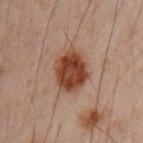Part of a total-body skin-imaging series; this lesion was reviewed on a skin check and was not flagged for biopsy.
A male patient, about 50 years old.
From the left upper arm.
This is a cross-polarized tile.
A 15 mm crop from a total-body photograph taken for skin-cancer surveillance.
An algorithmic analysis of the crop reported a footprint of about 14 mm². The software also gave a mean CIELAB color near L≈34 a*≈19 b*≈26, about 12 CIELAB-L* units darker than the surrounding skin, and a lesion-to-skin contrast of about 11.5 (normalized; higher = more distinct). And it measured border irregularity of about 1 on a 0–10 scale and a within-lesion color-variation index near 4/10.
The recorded lesion diameter is about 4.5 mm.